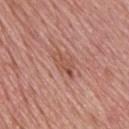Assessment: Captured during whole-body skin photography for melanoma surveillance; the lesion was not biopsied. Background: The subject is a male roughly 75 years of age. Located on the upper back. A 15 mm close-up tile from a total-body photography series done for melanoma screening.